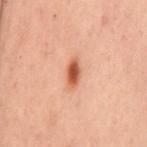A female subject, in their mid-50s.
A 15 mm close-up tile from a total-body photography series done for melanoma screening.
Located on the mid back.